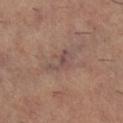Recorded during total-body skin imaging; not selected for excision or biopsy.
A female patient, approximately 60 years of age.
Located on the right lower leg.
A 15 mm close-up tile from a total-body photography series done for melanoma screening.
Captured under cross-polarized illumination.
Measured at roughly 3.5 mm in maximum diameter.